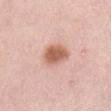Clinical impression:
Recorded during total-body skin imaging; not selected for excision or biopsy.
Image and clinical context:
Approximately 4 mm at its widest. A close-up tile cropped from a whole-body skin photograph, about 15 mm across. The lesion is on the abdomen. A female patient, aged around 60. Imaged with white-light lighting.